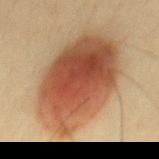Recorded during total-body skin imaging; not selected for excision or biopsy.
A lesion tile, about 15 mm wide, cut from a 3D total-body photograph.
The patient is a male in their 40s.
Longest diameter approximately 11 mm.
Imaged with cross-polarized lighting.
The lesion is on the mid back.